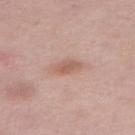A close-up tile cropped from a whole-body skin photograph, about 15 mm across.
Captured under white-light illumination.
Approximately 3 mm at its widest.
From the abdomen.
The subject is a male aged 73 to 77.